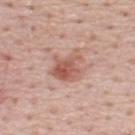illumination: white-light illumination
size: ≈4 mm
subject: male, aged 48–52
site: the upper back
image: ~15 mm crop, total-body skin-cancer survey